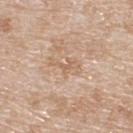{"biopsy_status": "not biopsied; imaged during a skin examination", "lesion_size": {"long_diameter_mm_approx": 4.0}, "image": {"source": "total-body photography crop", "field_of_view_mm": 15}, "patient": {"sex": "male", "age_approx": 80}, "site": "back"}